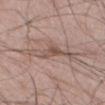Located on the left thigh. Automated image analysis of the tile measured a color-variation rating of about 4.5/10 and radial color variation of about 1. A male subject approximately 60 years of age. Captured under white-light illumination. A 15 mm crop from a total-body photograph taken for skin-cancer surveillance. The lesion's longest dimension is about 6 mm.